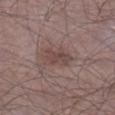A roughly 15 mm field-of-view crop from a total-body skin photograph.
The recorded lesion diameter is about 4 mm.
Imaged with white-light lighting.
An algorithmic analysis of the crop reported a mean CIELAB color near L≈44 a*≈17 b*≈19 and a normalized border contrast of about 6.5. And it measured a peripheral color-asymmetry measure near 1. The analysis additionally found an automated nevus-likeness rating near 5 out of 100 and a lesion-detection confidence of about 100/100.
Located on the right lower leg.
The patient is a male in their mid-60s.